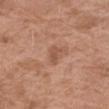Captured during whole-body skin photography for melanoma surveillance; the lesion was not biopsied.
A 15 mm crop from a total-body photograph taken for skin-cancer surveillance.
About 2.5 mm across.
Automated tile analysis of the lesion measured a lesion color around L≈52 a*≈22 b*≈31 in CIELAB and a lesion-to-skin contrast of about 6 (normalized; higher = more distinct). The software also gave a nevus-likeness score of about 0/100 and a detector confidence of about 100 out of 100 that the crop contains a lesion.
The lesion is on the right upper arm.
A female subject, aged 73–77.
This is a white-light tile.